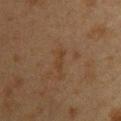Notes:
- site — the upper back
- acquisition — 15 mm crop, total-body photography
- automated lesion analysis — an average lesion color of about L≈31 a*≈15 b*≈26 (CIELAB), about 4 CIELAB-L* units darker than the surrounding skin, and a normalized lesion–skin contrast near 5
- subject — female, aged around 40